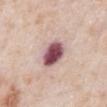- notes — no biopsy performed (imaged during a skin exam)
- site — the abdomen
- lighting — white-light illumination
- diameter — ≈4 mm
- patient — male, approximately 80 years of age
- imaging modality — 15 mm crop, total-body photography